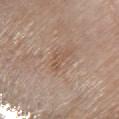Q: Was a biopsy performed?
A: no biopsy performed (imaged during a skin exam)
Q: How large is the lesion?
A: about 3.5 mm
Q: How was this image acquired?
A: 15 mm crop, total-body photography
Q: What lighting was used for the tile?
A: white-light
Q: Lesion location?
A: the upper back
Q: Patient demographics?
A: female, in their mid-60s